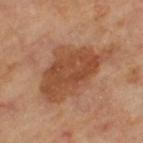Captured during whole-body skin photography for melanoma surveillance; the lesion was not biopsied. The total-body-photography lesion software estimated a footprint of about 30 mm² and a shape eccentricity near 0.8. It also reported a border-irregularity rating of about 4/10. And it measured a nevus-likeness score of about 5/100 and lesion-presence confidence of about 100/100. A female patient, in their mid- to late 50s. A 15 mm close-up tile from a total-body photography series done for melanoma screening. From the left thigh.